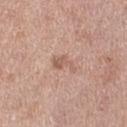Captured during whole-body skin photography for melanoma surveillance; the lesion was not biopsied.
The tile uses white-light illumination.
The lesion-visualizer software estimated an area of roughly 4 mm², an eccentricity of roughly 0.75, and a shape-asymmetry score of about 0.55 (0 = symmetric). And it measured a border-irregularity index near 6/10 and a within-lesion color-variation index near 2/10. And it measured a nevus-likeness score of about 5/100.
This image is a 15 mm lesion crop taken from a total-body photograph.
The lesion's longest dimension is about 3 mm.
The lesion is located on the leg.
A female subject, roughly 40 years of age.